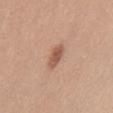Clinical impression:
Part of a total-body skin-imaging series; this lesion was reviewed on a skin check and was not flagged for biopsy.
Acquisition and patient details:
Approximately 3.5 mm at its widest. The lesion is on the front of the torso. The total-body-photography lesion software estimated an average lesion color of about L≈56 a*≈22 b*≈30 (CIELAB), about 11 CIELAB-L* units darker than the surrounding skin, and a lesion-to-skin contrast of about 7.5 (normalized; higher = more distinct). The software also gave an automated nevus-likeness rating near 95 out of 100 and a detector confidence of about 100 out of 100 that the crop contains a lesion. This is a white-light tile. A region of skin cropped from a whole-body photographic capture, roughly 15 mm wide. A male subject in their mid-30s.